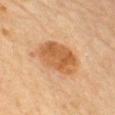Impression:
Recorded during total-body skin imaging; not selected for excision or biopsy.
Acquisition and patient details:
A 15 mm close-up extracted from a 3D total-body photography capture. The lesion-visualizer software estimated a footprint of about 17 mm², an eccentricity of roughly 0.8, and two-axis asymmetry of about 0.2. And it measured a classifier nevus-likeness of about 90/100 and lesion-presence confidence of about 100/100. A male subject aged 68 to 72. Located on the chest. This is a cross-polarized tile. Longest diameter approximately 6 mm.